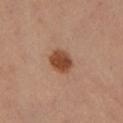The lesion was photographed on a routine skin check and not biopsied; there is no pathology result. A female subject aged around 55. Automated tile analysis of the lesion measured a footprint of about 7 mm² and a shape eccentricity near 0.5. Imaged with cross-polarized lighting. This image is a 15 mm lesion crop taken from a total-body photograph. Longest diameter approximately 3 mm. The lesion is located on the left lower leg.